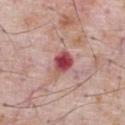follow-up: no biopsy performed (imaged during a skin exam); subject: male, aged approximately 70; image source: 15 mm crop, total-body photography; lesion diameter: ~3 mm (longest diameter); tile lighting: white-light; body site: the chest.